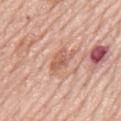Clinical impression:
The lesion was tiled from a total-body skin photograph and was not biopsied.
Clinical summary:
On the mid back. Approximately 3.5 mm at its widest. This is a white-light tile. The total-body-photography lesion software estimated a border-irregularity index near 4/10 and a color-variation rating of about 3.5/10. A lesion tile, about 15 mm wide, cut from a 3D total-body photograph. A male subject aged around 80.